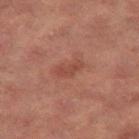Captured during whole-body skin photography for melanoma surveillance; the lesion was not biopsied.
Approximately 3 mm at its widest.
A 15 mm close-up extracted from a 3D total-body photography capture.
On the right thigh.
A female patient, aged approximately 70.
Captured under cross-polarized illumination.